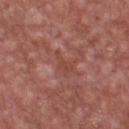The lesion was tiled from a total-body skin photograph and was not biopsied. The lesion's longest dimension is about 3.5 mm. An algorithmic analysis of the crop reported a shape eccentricity near 0.9. The analysis additionally found a mean CIELAB color near L≈45 a*≈26 b*≈26, a lesion–skin lightness drop of about 6, and a lesion-to-skin contrast of about 5 (normalized; higher = more distinct). The analysis additionally found border irregularity of about 5.5 on a 0–10 scale, a color-variation rating of about 1/10, and peripheral color asymmetry of about 0.5. It also reported a nevus-likeness score of about 0/100 and a lesion-detection confidence of about 55/100. The lesion is on the front of the torso. Imaged with white-light lighting. A close-up tile cropped from a whole-body skin photograph, about 15 mm across. A male patient approximately 65 years of age.